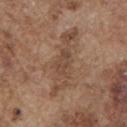<record>
<biopsy_status>not biopsied; imaged during a skin examination</biopsy_status>
<site>front of the torso</site>
<lesion_size>
  <long_diameter_mm_approx>3.5</long_diameter_mm_approx>
</lesion_size>
<image>
  <source>total-body photography crop</source>
  <field_of_view_mm>15</field_of_view_mm>
</image>
<lighting>white-light</lighting>
<patient>
  <sex>male</sex>
  <age_approx>75</age_approx>
</patient>
</record>